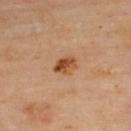Context:
The recorded lesion diameter is about 3 mm. From the upper back. This image is a 15 mm lesion crop taken from a total-body photograph. A female subject, aged around 60.